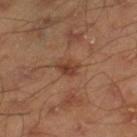Part of a total-body skin-imaging series; this lesion was reviewed on a skin check and was not flagged for biopsy. From the right lower leg. The patient is a male aged 43–47. The total-body-photography lesion software estimated a mean CIELAB color near L≈39 a*≈22 b*≈30, a lesion–skin lightness drop of about 9, and a normalized border contrast of about 7.5. It also reported a classifier nevus-likeness of about 75/100 and lesion-presence confidence of about 100/100. A region of skin cropped from a whole-body photographic capture, roughly 15 mm wide. This is a cross-polarized tile.